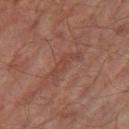workup = catalogued during a skin exam; not biopsied
patient = male, aged 63–67
acquisition = total-body-photography crop, ~15 mm field of view
location = the right thigh